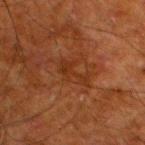Recorded during total-body skin imaging; not selected for excision or biopsy.
The lesion is on the leg.
A region of skin cropped from a whole-body photographic capture, roughly 15 mm wide.
A male patient approximately 80 years of age.